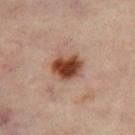Recorded during total-body skin imaging; not selected for excision or biopsy.
A region of skin cropped from a whole-body photographic capture, roughly 15 mm wide.
A female patient, aged approximately 55.
From the leg.